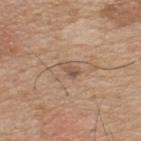Clinical impression: This lesion was catalogued during total-body skin photography and was not selected for biopsy. Context: A 15 mm close-up tile from a total-body photography series done for melanoma screening. The lesion is on the upper back. Captured under white-light illumination. The lesion's longest dimension is about 2.5 mm. The patient is a male about 75 years old. The total-body-photography lesion software estimated an area of roughly 2.5 mm², a shape eccentricity near 0.85, and a shape-asymmetry score of about 0.4 (0 = symmetric). The analysis additionally found a lesion–skin lightness drop of about 9. The software also gave a border-irregularity index near 3.5/10, internal color variation of about 2 on a 0–10 scale, and peripheral color asymmetry of about 0.5. And it measured an automated nevus-likeness rating near 0 out of 100 and a detector confidence of about 100 out of 100 that the crop contains a lesion.